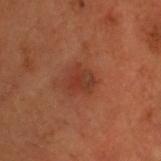Impression:
Recorded during total-body skin imaging; not selected for excision or biopsy.
Image and clinical context:
A 15 mm close-up tile from a total-body photography series done for melanoma screening. Located on the head or neck. The subject is a male approximately 50 years of age. This is a cross-polarized tile.